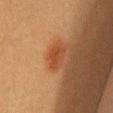This lesion was catalogued during total-body skin photography and was not selected for biopsy. From the chest. Cropped from a whole-body photographic skin survey; the tile spans about 15 mm. This is a cross-polarized tile. A female subject, aged 28 to 32. About 3 mm across.